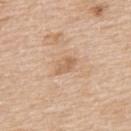| field | value |
|---|---|
| biopsy status | total-body-photography surveillance lesion; no biopsy |
| subject | male, aged 58–62 |
| anatomic site | the upper back |
| acquisition | ~15 mm crop, total-body skin-cancer survey |